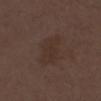Acquisition and patient details: From the chest. A lesion tile, about 15 mm wide, cut from a 3D total-body photograph. A female subject, aged around 50. About 4.5 mm across.